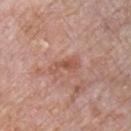biopsy status: imaged on a skin check; not biopsied